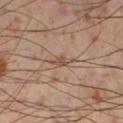Captured during whole-body skin photography for melanoma surveillance; the lesion was not biopsied. The tile uses cross-polarized illumination. This image is a 15 mm lesion crop taken from a total-body photograph. The lesion's longest dimension is about 2.5 mm. The lesion is located on the left lower leg. A male patient in their mid-50s.